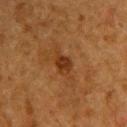Imaged during a routine full-body skin examination; the lesion was not biopsied and no histopathology is available. The subject is a male about 60 years old. Imaged with cross-polarized lighting. A 15 mm close-up tile from a total-body photography series done for melanoma screening. The recorded lesion diameter is about 2.5 mm. The lesion is located on the upper back. An algorithmic analysis of the crop reported about 8 CIELAB-L* units darker than the surrounding skin and a normalized lesion–skin contrast near 8.5. And it measured a border-irregularity index near 2.5/10 and a color-variation rating of about 1/10.